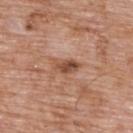Imaged during a routine full-body skin examination; the lesion was not biopsied and no histopathology is available.
The subject is a male about 60 years old.
A roughly 15 mm field-of-view crop from a total-body skin photograph.
This is a white-light tile.
An algorithmic analysis of the crop reported a border-irregularity index near 3/10 and a peripheral color-asymmetry measure near 2.5.
The lesion is on the upper back.